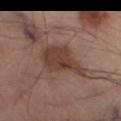| feature | finding |
|---|---|
| follow-up | total-body-photography surveillance lesion; no biopsy |
| imaging modality | ~15 mm crop, total-body skin-cancer survey |
| image-analysis metrics | lesion-presence confidence of about 100/100 |
| lesion diameter | ~6 mm (longest diameter) |
| body site | the leg |
| lighting | cross-polarized |